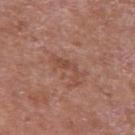The lesion was photographed on a routine skin check and not biopsied; there is no pathology result. The recorded lesion diameter is about 4.5 mm. A close-up tile cropped from a whole-body skin photograph, about 15 mm across. The subject is a male aged 63 to 67. The lesion is on the right upper arm.